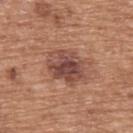A 15 mm close-up extracted from a 3D total-body photography capture.
The lesion is on the upper back.
The patient is a male aged 73 to 77.